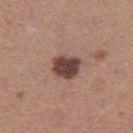{
  "lighting": "white-light",
  "patient": {
    "sex": "female",
    "age_approx": 35
  },
  "lesion_size": {
    "long_diameter_mm_approx": 3.0
  },
  "site": "right thigh",
  "image": {
    "source": "total-body photography crop",
    "field_of_view_mm": 15
  },
  "automated_metrics": {
    "area_mm2_approx": 7.5,
    "shape_asymmetry": 0.2
  }
}